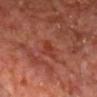Q: Is there a histopathology result?
A: imaged on a skin check; not biopsied
Q: Where on the body is the lesion?
A: the chest
Q: Patient demographics?
A: male, aged 63 to 67
Q: What is the imaging modality?
A: ~15 mm crop, total-body skin-cancer survey
Q: What lighting was used for the tile?
A: cross-polarized illumination
Q: How large is the lesion?
A: ~2.5 mm (longest diameter)